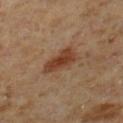Captured during whole-body skin photography for melanoma surveillance; the lesion was not biopsied. The lesion is on the left lower leg. Imaged with cross-polarized lighting. The total-body-photography lesion software estimated border irregularity of about 3 on a 0–10 scale, a within-lesion color-variation index near 2.5/10, and a peripheral color-asymmetry measure near 0.5. The patient is a male approximately 60 years of age. A 15 mm crop from a total-body photograph taken for skin-cancer surveillance.